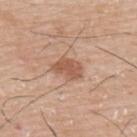notes: catalogued during a skin exam; not biopsied
body site: the upper back
patient: male, in their mid-70s
image: total-body-photography crop, ~15 mm field of view